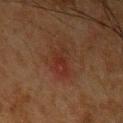| field | value |
|---|---|
| notes | no biopsy performed (imaged during a skin exam) |